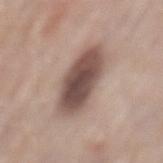The lesion was photographed on a routine skin check and not biopsied; there is no pathology result. Captured under white-light illumination. From the mid back. An algorithmic analysis of the crop reported a lesion area of about 18 mm² and a symmetry-axis asymmetry near 0.1. And it measured a lesion color around L≈49 a*≈17 b*≈21 in CIELAB and a lesion–skin lightness drop of about 17. Cropped from a total-body skin-imaging series; the visible field is about 15 mm. The subject is a male aged around 55.